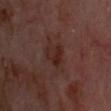Captured during whole-body skin photography for melanoma surveillance; the lesion was not biopsied. Automated tile analysis of the lesion measured a mean CIELAB color near L≈26 a*≈19 b*≈21, about 5 CIELAB-L* units darker than the surrounding skin, and a normalized border contrast of about 6.5. The analysis additionally found border irregularity of about 4 on a 0–10 scale and a peripheral color-asymmetry measure near 1.5. A subject in their mid-60s. A region of skin cropped from a whole-body photographic capture, roughly 15 mm wide. From the head or neck. The lesion's longest dimension is about 4 mm. Captured under cross-polarized illumination.